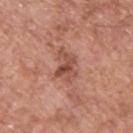This lesion was catalogued during total-body skin photography and was not selected for biopsy.
A male patient, aged 53 to 57.
A roughly 15 mm field-of-view crop from a total-body skin photograph.
The lesion is located on the upper back.